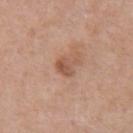workup: total-body-photography surveillance lesion; no biopsy | lesion size: ≈3 mm | subject: male, aged 68–72 | site: the chest | image source: ~15 mm crop, total-body skin-cancer survey.